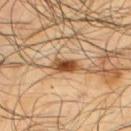follow-up: catalogued during a skin exam; not biopsied
lesion size: about 3.5 mm
anatomic site: the upper back
lighting: cross-polarized illumination
imaging modality: ~15 mm tile from a whole-body skin photo
patient: male, aged around 65